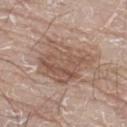Clinical impression: No biopsy was performed on this lesion — it was imaged during a full skin examination and was not determined to be concerning. Image and clinical context: A male patient in their 80s. This is a white-light tile. An algorithmic analysis of the crop reported a footprint of about 24 mm² and a shape eccentricity near 0.7. The software also gave an average lesion color of about L≈54 a*≈17 b*≈26 (CIELAB), about 10 CIELAB-L* units darker than the surrounding skin, and a normalized lesion–skin contrast near 7. The analysis additionally found an automated nevus-likeness rating near 60 out of 100. Located on the left leg. The lesion's longest dimension is about 6.5 mm. A lesion tile, about 15 mm wide, cut from a 3D total-body photograph.